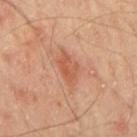The patient is a male in their mid-60s. Located on the mid back. This image is a 15 mm lesion crop taken from a total-body photograph. Captured under cross-polarized illumination. An algorithmic analysis of the crop reported an average lesion color of about L≈55 a*≈26 b*≈34 (CIELAB), about 8 CIELAB-L* units darker than the surrounding skin, and a normalized border contrast of about 6. The analysis additionally found internal color variation of about 3.5 on a 0–10 scale and a peripheral color-asymmetry measure near 1. And it measured a lesion-detection confidence of about 100/100.